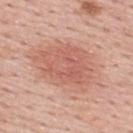Recorded during total-body skin imaging; not selected for excision or biopsy.
The tile uses white-light illumination.
A 15 mm crop from a total-body photograph taken for skin-cancer surveillance.
On the upper back.
Automated image analysis of the tile measured a nevus-likeness score of about 80/100 and a detector confidence of about 100 out of 100 that the crop contains a lesion.
The subject is a male in their mid-50s.